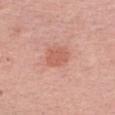workup: total-body-photography surveillance lesion; no biopsy | lesion size: ≈3 mm | imaging modality: ~15 mm crop, total-body skin-cancer survey | location: the arm | patient: female, aged 63 to 67 | automated lesion analysis: a footprint of about 5 mm², an eccentricity of roughly 0.6, and two-axis asymmetry of about 0.25; a lesion–skin lightness drop of about 8 and a normalized lesion–skin contrast near 5.5; a classifier nevus-likeness of about 70/100 and a detector confidence of about 100 out of 100 that the crop contains a lesion | tile lighting: white-light.